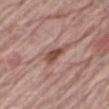Clinical impression:
Imaged during a routine full-body skin examination; the lesion was not biopsied and no histopathology is available.